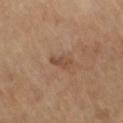The lesion was tiled from a total-body skin photograph and was not biopsied. This is a cross-polarized tile. Approximately 3 mm at its widest. A 15 mm crop from a total-body photograph taken for skin-cancer surveillance. The lesion is on the left leg. The lesion-visualizer software estimated an area of roughly 3 mm² and two-axis asymmetry of about 0.35. The analysis additionally found border irregularity of about 4 on a 0–10 scale and radial color variation of about 0. The analysis additionally found lesion-presence confidence of about 100/100. A male subject, approximately 65 years of age.